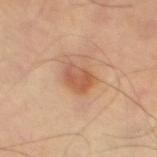Q: Was this lesion biopsied?
A: imaged on a skin check; not biopsied
Q: How was this image acquired?
A: 15 mm crop, total-body photography
Q: What is the anatomic site?
A: the leg
Q: What did automated image analysis measure?
A: a border-irregularity rating of about 2/10, a within-lesion color-variation index near 4/10, and radial color variation of about 1.5; an automated nevus-likeness rating near 85 out of 100 and a detector confidence of about 100 out of 100 that the crop contains a lesion
Q: How was the tile lit?
A: cross-polarized illumination